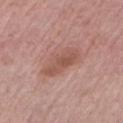The lesion was tiled from a total-body skin photograph and was not biopsied.
The subject is a male approximately 75 years of age.
On the left upper arm.
A 15 mm close-up extracted from a 3D total-body photography capture.